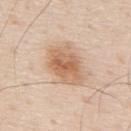Recorded during total-body skin imaging; not selected for excision or biopsy. A 15 mm crop from a total-body photograph taken for skin-cancer surveillance. A male subject in their 80s. The lesion is on the upper back.